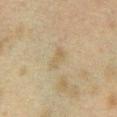* follow-up — imaged on a skin check; not biopsied
* anatomic site — the chest
* acquisition — ~15 mm crop, total-body skin-cancer survey
* subject — female, approximately 40 years of age